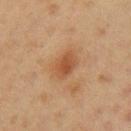Located on the left upper arm. A 15 mm close-up tile from a total-body photography series done for melanoma screening. The lesion's longest dimension is about 3 mm. Automated image analysis of the tile measured a mean CIELAB color near L≈44 a*≈21 b*≈32 and a normalized lesion–skin contrast near 7. The analysis additionally found a border-irregularity rating of about 2/10 and radial color variation of about 1. A female subject, aged around 20. The tile uses cross-polarized illumination.